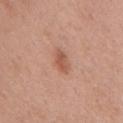Impression: Recorded during total-body skin imaging; not selected for excision or biopsy. Context: Measured at roughly 2.5 mm in maximum diameter. A 15 mm crop from a total-body photograph taken for skin-cancer surveillance. The lesion is located on the mid back. This is a white-light tile. The patient is a male aged around 70. Automated image analysis of the tile measured a footprint of about 3.5 mm², an outline eccentricity of about 0.8 (0 = round, 1 = elongated), and two-axis asymmetry of about 0.3. It also reported an average lesion color of about L≈55 a*≈25 b*≈31 (CIELAB) and about 10 CIELAB-L* units darker than the surrounding skin. And it measured a border-irregularity rating of about 3/10 and a peripheral color-asymmetry measure near 1. The analysis additionally found a nevus-likeness score of about 75/100 and lesion-presence confidence of about 100/100.